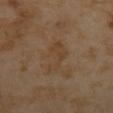Captured during whole-body skin photography for melanoma surveillance; the lesion was not biopsied.